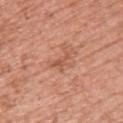<tbp_lesion>
  <biopsy_status>not biopsied; imaged during a skin examination</biopsy_status>
  <lesion_size>
    <long_diameter_mm_approx>2.5</long_diameter_mm_approx>
  </lesion_size>
  <automated_metrics>
    <vs_skin_darker_L>8.0</vs_skin_darker_L>
    <vs_skin_contrast_norm>6.0</vs_skin_contrast_norm>
  </automated_metrics>
  <site>upper back</site>
  <image>
    <source>total-body photography crop</source>
    <field_of_view_mm>15</field_of_view_mm>
  </image>
  <patient>
    <sex>female</sex>
    <age_approx>50</age_approx>
  </patient>
</tbp_lesion>